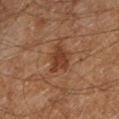{
  "biopsy_status": "not biopsied; imaged during a skin examination",
  "lighting": "cross-polarized",
  "image": {
    "source": "total-body photography crop",
    "field_of_view_mm": 15
  },
  "automated_metrics": {
    "cielab_L": 29,
    "cielab_a": 18,
    "cielab_b": 25,
    "vs_skin_darker_L": 7.0,
    "vs_skin_contrast_norm": 7.5,
    "border_irregularity_0_10": 3.5,
    "color_variation_0_10": 2.0,
    "peripheral_color_asymmetry": 0.5,
    "nevus_likeness_0_100": 20,
    "lesion_detection_confidence_0_100": 100
  },
  "site": "leg",
  "lesion_size": {
    "long_diameter_mm_approx": 3.5
  },
  "patient": {
    "sex": "male",
    "age_approx": 60
  }
}